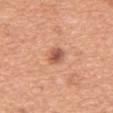Impression: Imaged during a routine full-body skin examination; the lesion was not biopsied and no histopathology is available. Clinical summary: A 15 mm crop from a total-body photograph taken for skin-cancer surveillance. The tile uses white-light illumination. The lesion-visualizer software estimated a lesion area of about 4 mm², a shape eccentricity near 0.8, and a shape-asymmetry score of about 0.2 (0 = symmetric). And it measured a classifier nevus-likeness of about 80/100 and lesion-presence confidence of about 100/100. A male patient aged 63–67. The lesion is on the abdomen. The recorded lesion diameter is about 3 mm.